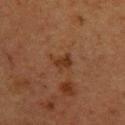Impression:
The lesion was photographed on a routine skin check and not biopsied; there is no pathology result.
Image and clinical context:
The lesion's longest dimension is about 2.5 mm. Imaged with cross-polarized lighting. On the upper back. An algorithmic analysis of the crop reported a lesion area of about 4 mm², a shape eccentricity near 0.55, and two-axis asymmetry of about 0.4. And it measured a mean CIELAB color near L≈29 a*≈19 b*≈27 and a normalized border contrast of about 7. The analysis additionally found an automated nevus-likeness rating near 0 out of 100 and a lesion-detection confidence of about 100/100. A 15 mm crop from a total-body photograph taken for skin-cancer surveillance. The patient is a female aged around 40.